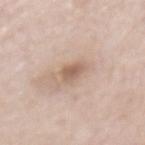Part of a total-body skin-imaging series; this lesion was reviewed on a skin check and was not flagged for biopsy. Located on the mid back. A male subject aged around 85 or older. A roughly 15 mm field-of-view crop from a total-body skin photograph.